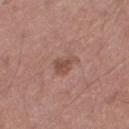biopsy_status: not biopsied; imaged during a skin examination
patient:
  sex: male
  age_approx: 60
site: right thigh
image:
  source: total-body photography crop
  field_of_view_mm: 15